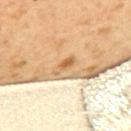Impression:
Imaged during a routine full-body skin examination; the lesion was not biopsied and no histopathology is available.
Background:
This image is a 15 mm lesion crop taken from a total-body photograph. From the upper back. A female patient, approximately 55 years of age.